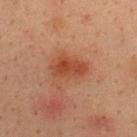The lesion was tiled from a total-body skin photograph and was not biopsied. The recorded lesion diameter is about 4.5 mm. The patient is a male about 35 years old. The lesion is located on the upper back. This image is a 15 mm lesion crop taken from a total-body photograph.